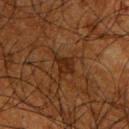biopsy status: total-body-photography surveillance lesion; no biopsy
acquisition: ~15 mm tile from a whole-body skin photo
image-analysis metrics: a footprint of about 4 mm², a shape eccentricity near 0.7, and a symmetry-axis asymmetry near 0.35; a border-irregularity index near 3.5/10, a within-lesion color-variation index near 2/10, and radial color variation of about 0.5; a nevus-likeness score of about 5/100 and a detector confidence of about 90 out of 100 that the crop contains a lesion
size: about 2.5 mm
site: the upper back
patient: male, aged 63 to 67
tile lighting: cross-polarized illumination